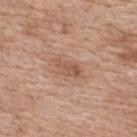The lesion was tiled from a total-body skin photograph and was not biopsied. Measured at roughly 3.5 mm in maximum diameter. From the upper back. A region of skin cropped from a whole-body photographic capture, roughly 15 mm wide. A female patient aged 38–42. Automated tile analysis of the lesion measured border irregularity of about 3.5 on a 0–10 scale, a color-variation rating of about 3/10, and radial color variation of about 1. The analysis additionally found a classifier nevus-likeness of about 5/100 and a lesion-detection confidence of about 100/100.